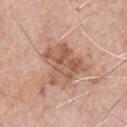  biopsy_status: not biopsied; imaged during a skin examination
  site: chest
  automated_metrics:
    area_mm2_approx: 17.0
    eccentricity: 0.45
    shape_asymmetry: 0.4
    cielab_L: 57
    cielab_a: 21
    cielab_b: 30
    vs_skin_darker_L: 10.0
    vs_skin_contrast_norm: 7.0
  lesion_size:
    long_diameter_mm_approx: 5.0
  lighting: white-light
  image:
    source: total-body photography crop
    field_of_view_mm: 15
  patient:
    sex: male
    age_approx: 70